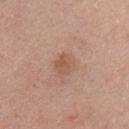follow-up — catalogued during a skin exam; not biopsied | site — the chest | patient — male, roughly 25 years of age | size — about 2.5 mm | imaging modality — ~15 mm tile from a whole-body skin photo | illumination — white-light illumination.